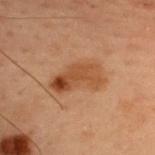Notes:
– workup · imaged on a skin check; not biopsied
– acquisition · ~15 mm crop, total-body skin-cancer survey
– illumination · cross-polarized illumination
– subject · female, aged approximately 40
– location · the upper back